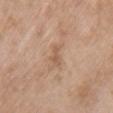Acquisition and patient details: The lesion is on the upper back. A female patient, in their 60s. A region of skin cropped from a whole-body photographic capture, roughly 15 mm wide.